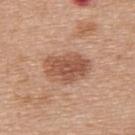notes = total-body-photography surveillance lesion; no biopsy | size = about 5.5 mm | tile lighting = white-light | acquisition = total-body-photography crop, ~15 mm field of view | patient = male, about 70 years old | body site = the back.